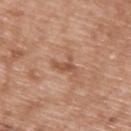Part of a total-body skin-imaging series; this lesion was reviewed on a skin check and was not flagged for biopsy. A male subject in their 50s. The lesion is on the back. The recorded lesion diameter is about 3 mm. A lesion tile, about 15 mm wide, cut from a 3D total-body photograph. Imaged with white-light lighting.